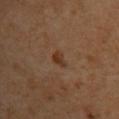Recorded during total-body skin imaging; not selected for excision or biopsy. Automated image analysis of the tile measured border irregularity of about 2.5 on a 0–10 scale, internal color variation of about 1 on a 0–10 scale, and a peripheral color-asymmetry measure near 0.5. And it measured an automated nevus-likeness rating near 70 out of 100 and a detector confidence of about 100 out of 100 that the crop contains a lesion. A female subject, aged 63 to 67. The recorded lesion diameter is about 2 mm. Imaged with cross-polarized lighting. A 15 mm close-up extracted from a 3D total-body photography capture. From the back.